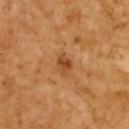{
  "biopsy_status": "not biopsied; imaged during a skin examination",
  "image": {
    "source": "total-body photography crop",
    "field_of_view_mm": 15
  },
  "site": "upper back",
  "patient": {
    "sex": "female",
    "age_approx": 55
  },
  "lesion_size": {
    "long_diameter_mm_approx": 3.0
  },
  "lighting": "cross-polarized"
}